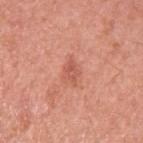Imaged during a routine full-body skin examination; the lesion was not biopsied and no histopathology is available. Approximately 2.5 mm at its widest. Captured under white-light illumination. A roughly 15 mm field-of-view crop from a total-body skin photograph. The lesion is on the left upper arm. A male patient, aged 53 to 57.